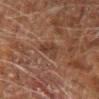Clinical summary:
Cropped from a total-body skin-imaging series; the visible field is about 15 mm. About 3 mm across. Imaged with cross-polarized lighting. A male subject, aged 58 to 62. The lesion is on the left arm. The total-body-photography lesion software estimated a mean CIELAB color near L≈28 a*≈15 b*≈21, about 5 CIELAB-L* units darker than the surrounding skin, and a normalized lesion–skin contrast near 5.5. The software also gave border irregularity of about 4 on a 0–10 scale and radial color variation of about 1.